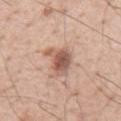No biopsy was performed on this lesion — it was imaged during a full skin examination and was not determined to be concerning.
The subject is a male aged 53–57.
The lesion-visualizer software estimated a mean CIELAB color near L≈57 a*≈21 b*≈27 and about 14 CIELAB-L* units darker than the surrounding skin. And it measured border irregularity of about 4.5 on a 0–10 scale and a within-lesion color-variation index near 4.5/10.
Cropped from a total-body skin-imaging series; the visible field is about 15 mm.
The tile uses white-light illumination.
Longest diameter approximately 4.5 mm.
On the back.